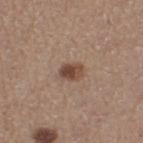<tbp_lesion>
<biopsy_status>not biopsied; imaged during a skin examination</biopsy_status>
<image>
  <source>total-body photography crop</source>
  <field_of_view_mm>15</field_of_view_mm>
</image>
<site>left thigh</site>
<lighting>white-light</lighting>
<patient>
  <sex>female</sex>
  <age_approx>45</age_approx>
</patient>
<automated_metrics>
  <eccentricity>0.65</eccentricity>
  <cielab_L>46</cielab_L>
  <cielab_a>18</cielab_a>
  <cielab_b>26</cielab_b>
  <vs_skin_darker_L>12.0</vs_skin_darker_L>
  <vs_skin_contrast_norm>9.0</vs_skin_contrast_norm>
  <border_irregularity_0_10>1.5</border_irregularity_0_10>
  <color_variation_0_10>5.5</color_variation_0_10>
  <peripheral_color_asymmetry>1.5</peripheral_color_asymmetry>
</automated_metrics>
<lesion_size>
  <long_diameter_mm_approx>2.5</long_diameter_mm_approx>
</lesion_size>
</tbp_lesion>